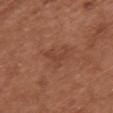Clinical impression:
Part of a total-body skin-imaging series; this lesion was reviewed on a skin check and was not flagged for biopsy.